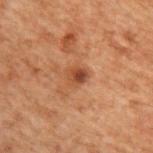workup: no biopsy performed (imaged during a skin exam)
location: the upper back
illumination: cross-polarized
lesion size: ≈2.5 mm
subject: male, aged 68–72
image source: ~15 mm crop, total-body skin-cancer survey